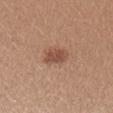The lesion was photographed on a routine skin check and not biopsied; there is no pathology result. The lesion is located on the left upper arm. A 15 mm close-up extracted from a 3D total-body photography capture. A female subject aged approximately 35.